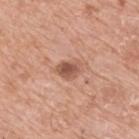Q: Was a biopsy performed?
A: imaged on a skin check; not biopsied
Q: What is the imaging modality?
A: ~15 mm tile from a whole-body skin photo
Q: Lesion location?
A: the upper back
Q: Who is the patient?
A: male, aged around 75